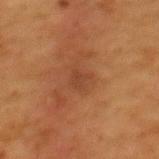Q: Was this lesion biopsied?
A: imaged on a skin check; not biopsied
Q: Who is the patient?
A: female, approximately 50 years of age
Q: What is the imaging modality?
A: ~15 mm crop, total-body skin-cancer survey
Q: Where on the body is the lesion?
A: the mid back
Q: How large is the lesion?
A: ≈3 mm